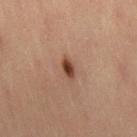workup = no biopsy performed (imaged during a skin exam); site = the right thigh; size = ≈2.5 mm; illumination = cross-polarized; patient = female, in their mid-40s; image = ~15 mm crop, total-body skin-cancer survey.